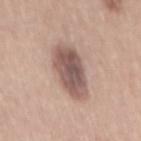Q: Was a biopsy performed?
A: catalogued during a skin exam; not biopsied
Q: What is the imaging modality?
A: 15 mm crop, total-body photography
Q: What are the patient's age and sex?
A: male, aged 53–57
Q: How was the tile lit?
A: white-light
Q: What is the anatomic site?
A: the mid back
Q: What is the lesion's diameter?
A: about 6.5 mm